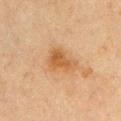biopsy status = no biopsy performed (imaged during a skin exam) | tile lighting = cross-polarized | acquisition = 15 mm crop, total-body photography | subject = male, aged around 65 | automated metrics = a border-irregularity index near 4/10, a color-variation rating of about 2.5/10, and peripheral color asymmetry of about 0.5; an automated nevus-likeness rating near 10 out of 100 and lesion-presence confidence of about 100/100 | size = about 4 mm | site = the right upper arm.